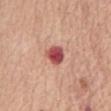Impression: Captured during whole-body skin photography for melanoma surveillance; the lesion was not biopsied. Image and clinical context: Cropped from a whole-body photographic skin survey; the tile spans about 15 mm. Automated tile analysis of the lesion measured a mean CIELAB color near L≈51 a*≈33 b*≈25, about 18 CIELAB-L* units darker than the surrounding skin, and a normalized border contrast of about 11.5. The analysis additionally found a lesion-detection confidence of about 100/100. The lesion is on the abdomen. A female patient, aged 53 to 57. Approximately 2.5 mm at its widest.